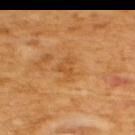Imaged during a routine full-body skin examination; the lesion was not biopsied and no histopathology is available.